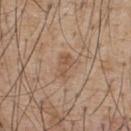Clinical impression:
Captured during whole-body skin photography for melanoma surveillance; the lesion was not biopsied.
Context:
A 15 mm crop from a total-body photograph taken for skin-cancer surveillance. The lesion-visualizer software estimated a nevus-likeness score of about 0/100 and a detector confidence of about 100 out of 100 that the crop contains a lesion. The lesion is on the upper back. This is a white-light tile. A male subject aged 53 to 57. The recorded lesion diameter is about 3 mm.